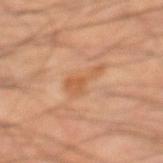This lesion was catalogued during total-body skin photography and was not selected for biopsy. The lesion is located on the left lower leg. The total-body-photography lesion software estimated an eccentricity of roughly 0.85 and two-axis asymmetry of about 0.65. It also reported a lesion color around L≈54 a*≈23 b*≈36 in CIELAB, about 7 CIELAB-L* units darker than the surrounding skin, and a normalized border contrast of about 6. Captured under cross-polarized illumination. Measured at roughly 4 mm in maximum diameter. A male subject approximately 45 years of age. A roughly 15 mm field-of-view crop from a total-body skin photograph.